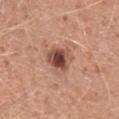Part of a total-body skin-imaging series; this lesion was reviewed on a skin check and was not flagged for biopsy. A male subject, in their mid-40s. A close-up tile cropped from a whole-body skin photograph, about 15 mm across. Located on the leg.